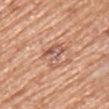Q: Automated lesion metrics?
A: a shape eccentricity near 0.8 and a symmetry-axis asymmetry near 0.55; a lesion color around L≈58 a*≈22 b*≈30 in CIELAB, roughly 10 lightness units darker than nearby skin, and a normalized lesion–skin contrast near 6.5; border irregularity of about 7 on a 0–10 scale and internal color variation of about 4.5 on a 0–10 scale; an automated nevus-likeness rating near 0 out of 100 and lesion-presence confidence of about 95/100
Q: What is the imaging modality?
A: ~15 mm tile from a whole-body skin photo
Q: Patient demographics?
A: female, aged 73–77
Q: What lighting was used for the tile?
A: white-light
Q: Lesion location?
A: the right upper arm